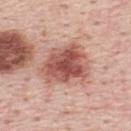No biopsy was performed on this lesion — it was imaged during a full skin examination and was not determined to be concerning. Automated tile analysis of the lesion measured a lesion area of about 22 mm² and a shape eccentricity near 0.7. The software also gave a lesion color around L≈55 a*≈26 b*≈26 in CIELAB, about 15 CIELAB-L* units darker than the surrounding skin, and a normalized border contrast of about 9.5. And it measured a nevus-likeness score of about 25/100 and a lesion-detection confidence of about 100/100. This is a white-light tile. A male subject in their mid- to late 40s. A lesion tile, about 15 mm wide, cut from a 3D total-body photograph. The recorded lesion diameter is about 6.5 mm. Located on the mid back.